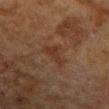Assessment: The lesion was photographed on a routine skin check and not biopsied; there is no pathology result. Context: About 3 mm across. The patient is a female in their 80s. On the right forearm. A region of skin cropped from a whole-body photographic capture, roughly 15 mm wide.